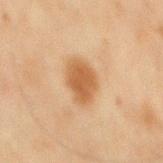| field | value |
|---|---|
| workup | catalogued during a skin exam; not biopsied |
| patient | male, in their mid-40s |
| lesion diameter | ~4.5 mm (longest diameter) |
| image-analysis metrics | border irregularity of about 2 on a 0–10 scale and peripheral color asymmetry of about 0.5; a lesion-detection confidence of about 100/100 |
| illumination | cross-polarized |
| imaging modality | total-body-photography crop, ~15 mm field of view |
| site | the mid back |